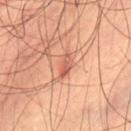workup = no biopsy performed (imaged during a skin exam) | tile lighting = cross-polarized | patient = male, aged 68–72 | image = ~15 mm tile from a whole-body skin photo | anatomic site = the leg.